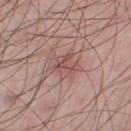Q: Was this lesion biopsied?
A: imaged on a skin check; not biopsied
Q: Lesion location?
A: the left lower leg
Q: How large is the lesion?
A: about 3 mm
Q: How was this image acquired?
A: total-body-photography crop, ~15 mm field of view
Q: Who is the patient?
A: male, roughly 45 years of age